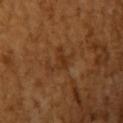Acquisition and patient details: The lesion is on the left upper arm. Cropped from a whole-body photographic skin survey; the tile spans about 15 mm. A female patient aged 53 to 57. Measured at roughly 3.5 mm in maximum diameter.